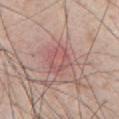Part of a total-body skin-imaging series; this lesion was reviewed on a skin check and was not flagged for biopsy. Captured under white-light illumination. The patient is a male aged 38 to 42. A region of skin cropped from a whole-body photographic capture, roughly 15 mm wide. Located on the chest. About 3 mm across.